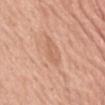biopsy_status: not biopsied; imaged during a skin examination
lighting: white-light
automated_metrics:
  area_mm2_approx: 6.0
  shape_asymmetry: 0.25
  border_irregularity_0_10: 2.5
  color_variation_0_10: 2.0
  peripheral_color_asymmetry: 0.5
  nevus_likeness_0_100: 0
  lesion_detection_confidence_0_100: 100
lesion_size:
  long_diameter_mm_approx: 4.0
image:
  source: total-body photography crop
  field_of_view_mm: 15
site: abdomen
patient:
  sex: female
  age_approx: 75